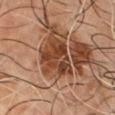Impression:
Recorded during total-body skin imaging; not selected for excision or biopsy.